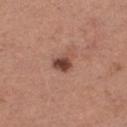{
  "image": {
    "source": "total-body photography crop",
    "field_of_view_mm": 15
  },
  "lighting": "white-light",
  "lesion_size": {
    "long_diameter_mm_approx": 2.5
  },
  "site": "left thigh",
  "patient": {
    "sex": "female",
    "age_approx": 30
  }
}